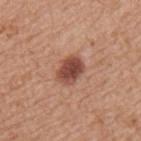  biopsy_status: not biopsied; imaged during a skin examination
  patient:
    sex: male
    age_approx: 65
  image:
    source: total-body photography crop
    field_of_view_mm: 15
  site: mid back
  automated_metrics:
    area_mm2_approx: 7.5
    eccentricity: 0.65
    nevus_likeness_0_100: 95
    lesion_detection_confidence_0_100: 100
  lesion_size:
    long_diameter_mm_approx: 3.5